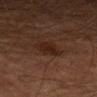biopsy_status: not biopsied; imaged during a skin examination
patient:
  sex: male
  age_approx: 60
site: left forearm
lesion_size:
  long_diameter_mm_approx: 3.5
lighting: cross-polarized
image:
  source: total-body photography crop
  field_of_view_mm: 15